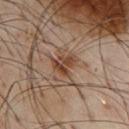A lesion tile, about 15 mm wide, cut from a 3D total-body photograph.
A male patient, aged 53 to 57.
Measured at roughly 3.5 mm in maximum diameter.
On the front of the torso.